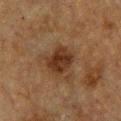{"biopsy_status": "not biopsied; imaged during a skin examination", "patient": {"sex": "female", "age_approx": 55}, "site": "chest", "automated_metrics": {"cielab_L": 27, "cielab_a": 17, "cielab_b": 26, "vs_skin_darker_L": 10.0, "vs_skin_contrast_norm": 10.0, "border_irregularity_0_10": 2.0}, "image": {"source": "total-body photography crop", "field_of_view_mm": 15}}